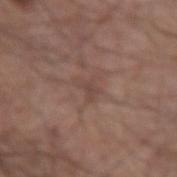Clinical impression:
This lesion was catalogued during total-body skin photography and was not selected for biopsy.
Background:
Longest diameter approximately 3 mm. This is a white-light tile. A male subject, aged 63–67. From the left upper arm. A lesion tile, about 15 mm wide, cut from a 3D total-body photograph.